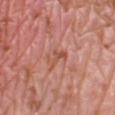  biopsy_status: not biopsied; imaged during a skin examination
  patient:
    sex: female
    age_approx: 40
  site: leg
  lesion_size:
    long_diameter_mm_approx: 3.0
  image:
    source: total-body photography crop
    field_of_view_mm: 15